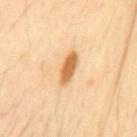  biopsy_status: not biopsied; imaged during a skin examination
  automated_metrics:
    area_mm2_approx: 5.0
    shape_asymmetry: 0.15
    cielab_L: 66
    cielab_a: 22
    cielab_b: 45
    vs_skin_darker_L: 16.0
    vs_skin_contrast_norm: 10.0
    border_irregularity_0_10: 2.0
    peripheral_color_asymmetry: 0.5
  patient:
    sex: male
    age_approx: 50
  site: mid back
  lighting: cross-polarized
  image:
    source: total-body photography crop
    field_of_view_mm: 15